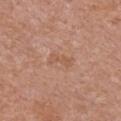Notes:
• follow-up: imaged on a skin check; not biopsied
• location: the chest
• acquisition: ~15 mm crop, total-body skin-cancer survey
• patient: female, aged approximately 50